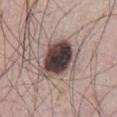Q: Is there a histopathology result?
A: total-body-photography surveillance lesion; no biopsy
Q: What kind of image is this?
A: ~15 mm crop, total-body skin-cancer survey
Q: How was the tile lit?
A: white-light illumination
Q: What is the anatomic site?
A: the abdomen
Q: Patient demographics?
A: male, aged around 65
Q: How large is the lesion?
A: ≈5.5 mm
Q: What did automated image analysis measure?
A: a footprint of about 17 mm² and a shape-asymmetry score of about 0.25 (0 = symmetric); a border-irregularity index near 3/10, a color-variation rating of about 9/10, and radial color variation of about 2.5; lesion-presence confidence of about 100/100